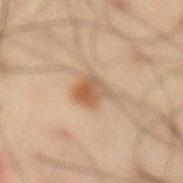The recorded lesion diameter is about 3 mm. The lesion-visualizer software estimated a footprint of about 6.5 mm², an outline eccentricity of about 0.45 (0 = round, 1 = elongated), and a symmetry-axis asymmetry near 0.2. The analysis additionally found a lesion color around L≈44 a*≈15 b*≈27 in CIELAB, roughly 8 lightness units darker than nearby skin, and a normalized lesion–skin contrast near 7.5. It also reported a border-irregularity index near 2.5/10 and a color-variation rating of about 5.5/10. Located on the abdomen. Cropped from a total-body skin-imaging series; the visible field is about 15 mm. A male subject aged around 60. This is a cross-polarized tile.